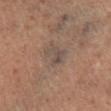This lesion was catalogued during total-body skin photography and was not selected for biopsy. Located on the left lower leg. Captured under cross-polarized illumination. Measured at roughly 3 mm in maximum diameter. A female patient, approximately 50 years of age. A 15 mm close-up extracted from a 3D total-body photography capture.